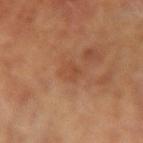A lesion tile, about 15 mm wide, cut from a 3D total-body photograph.
Automated tile analysis of the lesion measured a border-irregularity rating of about 3/10, internal color variation of about 1.5 on a 0–10 scale, and peripheral color asymmetry of about 0.5. The software also gave a detector confidence of about 100 out of 100 that the crop contains a lesion.
A female subject, aged 63–67.
The lesion is on the left arm.
Captured under cross-polarized illumination.
The recorded lesion diameter is about 2.5 mm.